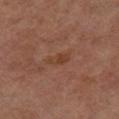Q: Was this lesion biopsied?
A: no biopsy performed (imaged during a skin exam)
Q: Lesion location?
A: the right thigh
Q: What are the patient's age and sex?
A: female, aged 58–62
Q: Lesion size?
A: about 3 mm
Q: What lighting was used for the tile?
A: cross-polarized
Q: Automated lesion metrics?
A: an area of roughly 4 mm² and a shape eccentricity near 0.85; a border-irregularity index near 3/10, a within-lesion color-variation index near 1.5/10, and radial color variation of about 0.5; a classifier nevus-likeness of about 0/100 and lesion-presence confidence of about 100/100
Q: What kind of image is this?
A: ~15 mm crop, total-body skin-cancer survey